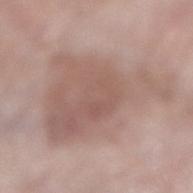biopsy_status: not biopsied; imaged during a skin examination
patient:
  sex: female
  age_approx: 70
image:
  source: total-body photography crop
  field_of_view_mm: 15
site: right lower leg